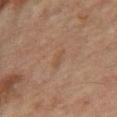The lesion is on the right thigh.
The patient is a female about 55 years old.
Imaged with cross-polarized lighting.
The lesion's longest dimension is about 3 mm.
Cropped from a total-body skin-imaging series; the visible field is about 15 mm.
Automated tile analysis of the lesion measured a footprint of about 3 mm² and two-axis asymmetry of about 0.3. The analysis additionally found a border-irregularity rating of about 3/10, a color-variation rating of about 0.5/10, and radial color variation of about 0.